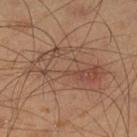follow-up — total-body-photography surveillance lesion; no biopsy
patient — male, aged 38 to 42
acquisition — 15 mm crop, total-body photography
site — the left lower leg
image-analysis metrics — a lesion area of about 25 mm², an outline eccentricity of about 0.9 (0 = round, 1 = elongated), and a symmetry-axis asymmetry near 0.35; a border-irregularity index near 5.5/10; a nevus-likeness score of about 5/100 and lesion-presence confidence of about 100/100
lesion size — about 8.5 mm
lighting — cross-polarized